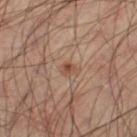The lesion was photographed on a routine skin check and not biopsied; there is no pathology result. Cropped from a whole-body photographic skin survey; the tile spans about 15 mm. The total-body-photography lesion software estimated a border-irregularity index near 2.5/10, internal color variation of about 1 on a 0–10 scale, and a peripheral color-asymmetry measure near 0. The patient is a male aged around 65. About 1.5 mm across. This is a cross-polarized tile. Located on the left thigh.